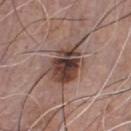workup: total-body-photography surveillance lesion; no biopsy
image: ~15 mm tile from a whole-body skin photo
patient: male, about 75 years old
body site: the chest